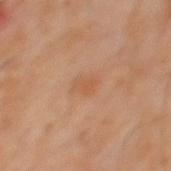Q: What is the anatomic site?
A: the mid back
Q: What kind of image is this?
A: total-body-photography crop, ~15 mm field of view
Q: Patient demographics?
A: male, about 60 years old
Q: What did automated image analysis measure?
A: peripheral color asymmetry of about 0.5
Q: Lesion size?
A: ~2.5 mm (longest diameter)